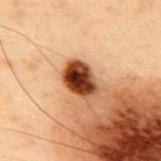Findings:
- notes · imaged on a skin check; not biopsied
- imaging modality · ~15 mm tile from a whole-body skin photo
- body site · the upper back
- subject · male, in their mid- to late 50s
- lesion diameter · ~4 mm (longest diameter)